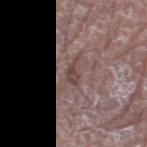Impression:
Recorded during total-body skin imaging; not selected for excision or biopsy.
Acquisition and patient details:
The total-body-photography lesion software estimated a lesion area of about 3 mm², an outline eccentricity of about 0.85 (0 = round, 1 = elongated), and a shape-asymmetry score of about 0.55 (0 = symmetric). And it measured a mean CIELAB color near L≈43 a*≈18 b*≈19, a lesion–skin lightness drop of about 8, and a normalized border contrast of about 7. It also reported a color-variation rating of about 2/10 and a peripheral color-asymmetry measure near 1. The software also gave a classifier nevus-likeness of about 0/100 and lesion-presence confidence of about 55/100. A region of skin cropped from a whole-body photographic capture, roughly 15 mm wide. A male patient in their mid-70s. The tile uses white-light illumination. On the left lower leg. Approximately 3 mm at its widest.